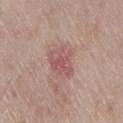Imaged during a routine full-body skin examination; the lesion was not biopsied and no histopathology is available. The lesion-visualizer software estimated a footprint of about 9.5 mm², an outline eccentricity of about 0.7 (0 = round, 1 = elongated), and two-axis asymmetry of about 0.3. The software also gave a mean CIELAB color near L≈54 a*≈23 b*≈20 and roughly 8 lightness units darker than nearby skin. The analysis additionally found a classifier nevus-likeness of about 5/100 and a lesion-detection confidence of about 100/100. The lesion is located on the right thigh. This is a white-light tile. This image is a 15 mm lesion crop taken from a total-body photograph. A male subject in their mid- to late 60s.